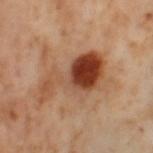{
  "biopsy_status": "not biopsied; imaged during a skin examination",
  "patient": {
    "sex": "female",
    "age_approx": 55
  },
  "image": {
    "source": "total-body photography crop",
    "field_of_view_mm": 15
  },
  "site": "left thigh",
  "automated_metrics": {
    "area_mm2_approx": 19.0,
    "eccentricity": 0.9,
    "shape_asymmetry": 0.5,
    "cielab_L": 46,
    "cielab_a": 25,
    "cielab_b": 34,
    "vs_skin_darker_L": 15.0,
    "vs_skin_contrast_norm": 10.5,
    "border_irregularity_0_10": 7.0,
    "nevus_likeness_0_100": 70,
    "lesion_detection_confidence_0_100": 100
  },
  "lighting": "cross-polarized"
}